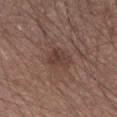– workup — no biopsy performed (imaged during a skin exam)
– site — the right upper arm
– image — total-body-photography crop, ~15 mm field of view
– subject — male, aged 53–57